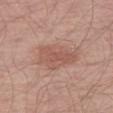Q: Was a biopsy performed?
A: total-body-photography surveillance lesion; no biopsy
Q: What is the lesion's diameter?
A: ~5 mm (longest diameter)
Q: Where on the body is the lesion?
A: the right thigh
Q: Who is the patient?
A: male, aged 63–67
Q: What is the imaging modality?
A: 15 mm crop, total-body photography
Q: Illumination type?
A: white-light illumination